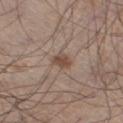Impression: No biopsy was performed on this lesion — it was imaged during a full skin examination and was not determined to be concerning. Acquisition and patient details: The recorded lesion diameter is about 2.5 mm. A 15 mm crop from a total-body photograph taken for skin-cancer surveillance. Imaged with white-light lighting. The subject is a male approximately 60 years of age. From the right thigh. Automated tile analysis of the lesion measured a lesion area of about 3.5 mm², an eccentricity of roughly 0.85, and two-axis asymmetry of about 0.25. It also reported a border-irregularity index near 2.5/10, a within-lesion color-variation index near 2/10, and a peripheral color-asymmetry measure near 1.